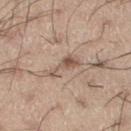Q: Is there a histopathology result?
A: total-body-photography surveillance lesion; no biopsy
Q: Illumination type?
A: white-light
Q: Lesion size?
A: about 4 mm
Q: Automated lesion metrics?
A: a nevus-likeness score of about 20/100 and lesion-presence confidence of about 95/100
Q: What is the imaging modality?
A: ~15 mm crop, total-body skin-cancer survey
Q: What are the patient's age and sex?
A: male, aged approximately 55
Q: Lesion location?
A: the left thigh